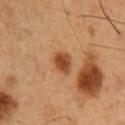Recorded during total-body skin imaging; not selected for excision or biopsy.
A male subject, aged around 50.
A region of skin cropped from a whole-body photographic capture, roughly 15 mm wide.
The lesion is located on the chest.